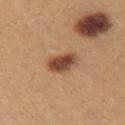No biopsy was performed on this lesion — it was imaged during a full skin examination and was not determined to be concerning.
A female patient in their mid- to late 20s.
A 15 mm crop from a total-body photograph taken for skin-cancer surveillance.
The lesion is on the chest.
The lesion's longest dimension is about 4 mm.
Captured under white-light illumination.